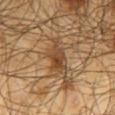notes: catalogued during a skin exam; not biopsied | location: the mid back | patient: male, about 65 years old | image: ~15 mm crop, total-body skin-cancer survey.